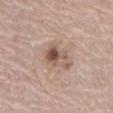Q: Is there a histopathology result?
A: no biopsy performed (imaged during a skin exam)
Q: Patient demographics?
A: female, roughly 65 years of age
Q: What is the lesion's diameter?
A: about 4.5 mm
Q: What is the imaging modality?
A: 15 mm crop, total-body photography
Q: What did automated image analysis measure?
A: an area of roughly 8.5 mm², a shape eccentricity near 0.8, and a shape-asymmetry score of about 0.35 (0 = symmetric)
Q: Lesion location?
A: the mid back
Q: How was the tile lit?
A: white-light